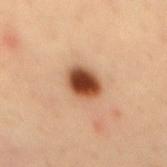Q: Was a biopsy performed?
A: total-body-photography surveillance lesion; no biopsy
Q: Illumination type?
A: cross-polarized illumination
Q: What kind of image is this?
A: 15 mm crop, total-body photography
Q: Lesion location?
A: the mid back
Q: What are the patient's age and sex?
A: male, aged 33–37
Q: What is the lesion's diameter?
A: ~3.5 mm (longest diameter)